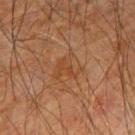The recorded lesion diameter is about 3.5 mm.
From the right forearm.
A region of skin cropped from a whole-body photographic capture, roughly 15 mm wide.
A male patient, about 65 years old.
This is a cross-polarized tile.